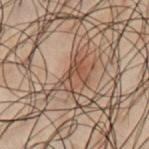Assessment: Imaged during a routine full-body skin examination; the lesion was not biopsied and no histopathology is available. Acquisition and patient details: Longest diameter approximately 3.5 mm. Cropped from a whole-body photographic skin survey; the tile spans about 15 mm. Located on the chest. The tile uses cross-polarized illumination. The patient is a male roughly 50 years of age. Automated tile analysis of the lesion measured an area of roughly 4.5 mm². It also reported a mean CIELAB color near L≈36 a*≈15 b*≈23, about 7 CIELAB-L* units darker than the surrounding skin, and a normalized border contrast of about 7. The software also gave border irregularity of about 7.5 on a 0–10 scale and a peripheral color-asymmetry measure near 0.5. The software also gave a nevus-likeness score of about 65/100 and a detector confidence of about 90 out of 100 that the crop contains a lesion.